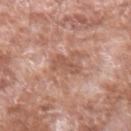| feature | finding |
|---|---|
| workup | no biopsy performed (imaged during a skin exam) |
| diameter | about 3.5 mm |
| subject | male, approximately 60 years of age |
| lighting | white-light |
| acquisition | ~15 mm crop, total-body skin-cancer survey |
| automated metrics | a footprint of about 5 mm², an eccentricity of roughly 0.85, and a shape-asymmetry score of about 0.3 (0 = symmetric); a within-lesion color-variation index near 1.5/10 and peripheral color asymmetry of about 0.5; a detector confidence of about 100 out of 100 that the crop contains a lesion |
| location | the left forearm |